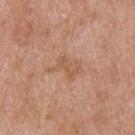Findings:
* patient — male, about 65 years old
* site — the upper back
* image source — 15 mm crop, total-body photography